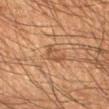About 2.5 mm across. This is a cross-polarized tile. A lesion tile, about 15 mm wide, cut from a 3D total-body photograph. A male patient, in their mid- to late 50s. On the left forearm. The total-body-photography lesion software estimated a border-irregularity index near 4.5/10, a within-lesion color-variation index near 2/10, and peripheral color asymmetry of about 0.5. And it measured a nevus-likeness score of about 0/100 and lesion-presence confidence of about 100/100.